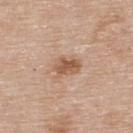Impression:
No biopsy was performed on this lesion — it was imaged during a full skin examination and was not determined to be concerning.
Background:
A close-up tile cropped from a whole-body skin photograph, about 15 mm across. This is a white-light tile. A female patient in their mid-60s. The lesion is located on the back. The recorded lesion diameter is about 3 mm. An algorithmic analysis of the crop reported a mean CIELAB color near L≈56 a*≈20 b*≈32, about 11 CIELAB-L* units darker than the surrounding skin, and a normalized lesion–skin contrast near 8. The software also gave a nevus-likeness score of about 40/100 and a detector confidence of about 100 out of 100 that the crop contains a lesion.